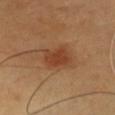Impression:
Imaged during a routine full-body skin examination; the lesion was not biopsied and no histopathology is available.
Acquisition and patient details:
The lesion's longest dimension is about 3.5 mm. This image is a 15 mm lesion crop taken from a total-body photograph. An algorithmic analysis of the crop reported an outline eccentricity of about 0.7 (0 = round, 1 = elongated) and a symmetry-axis asymmetry near 0.2. The software also gave radial color variation of about 1. The software also gave a nevus-likeness score of about 95/100. On the chest. The subject is a male aged around 45.